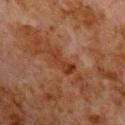Captured during whole-body skin photography for melanoma surveillance; the lesion was not biopsied.
The lesion is on the chest.
A male patient, aged 78 to 82.
A roughly 15 mm field-of-view crop from a total-body skin photograph.
This is a cross-polarized tile.